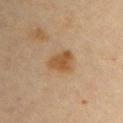Assessment:
This lesion was catalogued during total-body skin photography and was not selected for biopsy.
Acquisition and patient details:
On the chest. About 3.5 mm across. Cropped from a whole-body photographic skin survey; the tile spans about 15 mm. Automated image analysis of the tile measured a border-irregularity index near 2.5/10 and a within-lesion color-variation index near 3/10. The analysis additionally found an automated nevus-likeness rating near 85 out of 100 and a detector confidence of about 100 out of 100 that the crop contains a lesion. The tile uses cross-polarized illumination. A female patient, roughly 45 years of age.